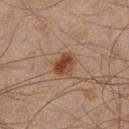workup — catalogued during a skin exam; not biopsied
body site — the leg
patient — male, aged 43–47
illumination — cross-polarized illumination
imaging modality — total-body-photography crop, ~15 mm field of view